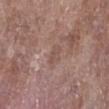workup — no biopsy performed (imaged during a skin exam) | TBP lesion metrics — a within-lesion color-variation index near 1/10 and a peripheral color-asymmetry measure near 0.5; a nevus-likeness score of about 0/100 and a detector confidence of about 95 out of 100 that the crop contains a lesion | subject — female, aged around 75 | body site — the right lower leg | imaging modality — total-body-photography crop, ~15 mm field of view.